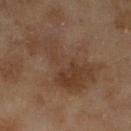Assessment:
The lesion was tiled from a total-body skin photograph and was not biopsied.